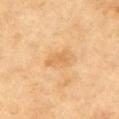biopsy status: total-body-photography surveillance lesion; no biopsy | patient: male, roughly 70 years of age | anatomic site: the left upper arm | lesion diameter: ≈3 mm | imaging modality: ~15 mm tile from a whole-body skin photo.